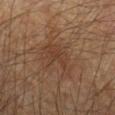Assessment:
Recorded during total-body skin imaging; not selected for excision or biopsy.
Clinical summary:
Measured at roughly 4 mm in maximum diameter. The tile uses cross-polarized illumination. A 15 mm crop from a total-body photograph taken for skin-cancer surveillance. The patient is a male aged around 60. The lesion is on the right forearm.